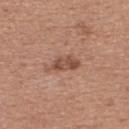Context: The lesion is located on the upper back. Approximately 4 mm at its widest. A female patient, aged 33 to 37. A region of skin cropped from a whole-body photographic capture, roughly 15 mm wide.